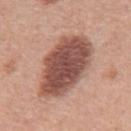No biopsy was performed on this lesion — it was imaged during a full skin examination and was not determined to be concerning. The lesion is on the mid back. Approximately 8 mm at its widest. A male patient, in their 70s. A 15 mm close-up extracted from a 3D total-body photography capture.